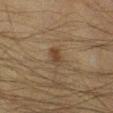Assessment: The lesion was photographed on a routine skin check and not biopsied; there is no pathology result. Context: Located on the right lower leg. A 15 mm close-up extracted from a 3D total-body photography capture. Captured under cross-polarized illumination. A male patient approximately 50 years of age.